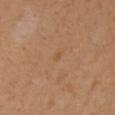workup — catalogued during a skin exam; not biopsied
image — total-body-photography crop, ~15 mm field of view
subject — female, approximately 40 years of age
location — the left upper arm
automated lesion analysis — a lesion area of about 1 mm², an outline eccentricity of about 0.6 (0 = round, 1 = elongated), and a symmetry-axis asymmetry near 0.3; border irregularity of about 2.5 on a 0–10 scale